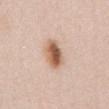Clinical summary: Measured at roughly 4 mm in maximum diameter. The lesion is located on the front of the torso. A female patient aged 38 to 42. The tile uses white-light illumination. A lesion tile, about 15 mm wide, cut from a 3D total-body photograph. The lesion-visualizer software estimated a lesion area of about 9 mm², a shape eccentricity near 0.7, and a symmetry-axis asymmetry near 0.15. The analysis additionally found an average lesion color of about L≈61 a*≈19 b*≈31 (CIELAB) and a normalized lesion–skin contrast near 9.5. The software also gave border irregularity of about 1.5 on a 0–10 scale, a within-lesion color-variation index near 8.5/10, and a peripheral color-asymmetry measure near 3. And it measured a detector confidence of about 100 out of 100 that the crop contains a lesion.